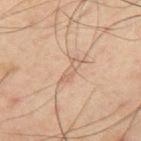{"biopsy_status": "not biopsied; imaged during a skin examination", "site": "mid back", "patient": {"sex": "male", "age_approx": 60}, "image": {"source": "total-body photography crop", "field_of_view_mm": 15}}